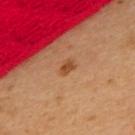Clinical impression: Captured during whole-body skin photography for melanoma surveillance; the lesion was not biopsied. Context: The patient is a female in their mid-30s. Cropped from a whole-body photographic skin survey; the tile spans about 15 mm. From the upper back. Captured under cross-polarized illumination. About 2 mm across.